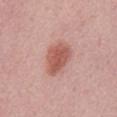A 15 mm close-up extracted from a 3D total-body photography capture. Located on the front of the torso. The lesion's longest dimension is about 4.5 mm. Imaged with white-light lighting. A male subject roughly 60 years of age.